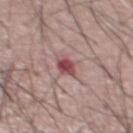No biopsy was performed on this lesion — it was imaged during a full skin examination and was not determined to be concerning. This is a white-light tile. The patient is a male aged approximately 55. A 15 mm crop from a total-body photograph taken for skin-cancer surveillance. The total-body-photography lesion software estimated an eccentricity of roughly 0.75 and a symmetry-axis asymmetry near 0.2. And it measured a classifier nevus-likeness of about 5/100 and lesion-presence confidence of about 100/100. On the chest.